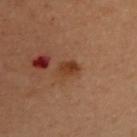Q: Is there a histopathology result?
A: catalogued during a skin exam; not biopsied
Q: What kind of image is this?
A: ~15 mm crop, total-body skin-cancer survey
Q: What is the anatomic site?
A: the chest
Q: Lesion size?
A: about 2.5 mm
Q: Patient demographics?
A: female, roughly 50 years of age